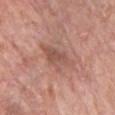- follow-up · total-body-photography surveillance lesion; no biopsy